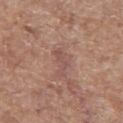The lesion was photographed on a routine skin check and not biopsied; there is no pathology result. Automated image analysis of the tile measured a classifier nevus-likeness of about 0/100. A female patient, approximately 75 years of age. This is a white-light tile. On the right thigh. About 3 mm across. A lesion tile, about 15 mm wide, cut from a 3D total-body photograph.